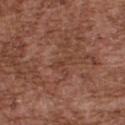Imaged during a routine full-body skin examination; the lesion was not biopsied and no histopathology is available. The lesion is located on the upper back. A male subject roughly 75 years of age. This image is a 15 mm lesion crop taken from a total-body photograph.